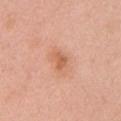From the left upper arm.
Approximately 2.5 mm at its widest.
A female patient about 40 years old.
Imaged with white-light lighting.
A 15 mm crop from a total-body photograph taken for skin-cancer surveillance.